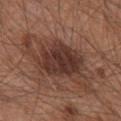- lighting — white-light illumination
- location — the chest
- subject — male, in their mid- to late 60s
- image source — total-body-photography crop, ~15 mm field of view